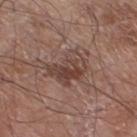The lesion was tiled from a total-body skin photograph and was not biopsied.
Measured at roughly 4.5 mm in maximum diameter.
On the left lower leg.
A lesion tile, about 15 mm wide, cut from a 3D total-body photograph.
A male subject about 60 years old.
This is a white-light tile.